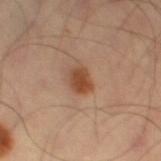follow-up: imaged on a skin check; not biopsied, illumination: cross-polarized, body site: the left thigh, image source: ~15 mm tile from a whole-body skin photo, automated lesion analysis: roughly 12 lightness units darker than nearby skin and a lesion-to-skin contrast of about 9.5 (normalized; higher = more distinct); an automated nevus-likeness rating near 95 out of 100 and a lesion-detection confidence of about 100/100.